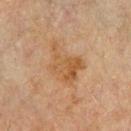Clinical impression: No biopsy was performed on this lesion — it was imaged during a full skin examination and was not determined to be concerning. Image and clinical context: A close-up tile cropped from a whole-body skin photograph, about 15 mm across. A male patient, aged 68 to 72. Approximately 5 mm at its widest. Automated tile analysis of the lesion measured a lesion area of about 13 mm² and a shape-asymmetry score of about 0.35 (0 = symmetric). And it measured a mean CIELAB color near L≈45 a*≈17 b*≈32 and roughly 6 lightness units darker than nearby skin. From the chest.